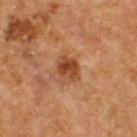lighting: cross-polarized
acquisition: ~15 mm crop, total-body skin-cancer survey
diameter: ≈2.5 mm
subject: male, roughly 50 years of age
location: the upper back
automated lesion analysis: a shape eccentricity near 0.6 and a symmetry-axis asymmetry near 0.2; a border-irregularity index near 2/10, a color-variation rating of about 4/10, and radial color variation of about 1.5; lesion-presence confidence of about 100/100